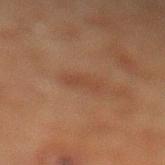No biopsy was performed on this lesion — it was imaged during a full skin examination and was not determined to be concerning.
Captured under cross-polarized illumination.
Automated tile analysis of the lesion measured about 5 CIELAB-L* units darker than the surrounding skin and a normalized lesion–skin contrast near 5.
A roughly 15 mm field-of-view crop from a total-body skin photograph.
On the left lower leg.
A male subject, about 60 years old.
The recorded lesion diameter is about 3 mm.